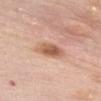Located on the chest. The patient is a female aged approximately 60. This image is a 15 mm lesion crop taken from a total-body photograph.